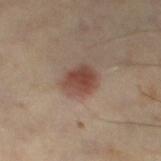biopsy_status: not biopsied; imaged during a skin examination